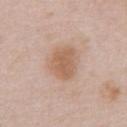Background:
About 4 mm across. A male patient approximately 55 years of age. The lesion is on the abdomen. Captured under white-light illumination. The total-body-photography lesion software estimated a lesion area of about 10 mm², an outline eccentricity of about 0.65 (0 = round, 1 = elongated), and a shape-asymmetry score of about 0.2 (0 = symmetric). It also reported an automated nevus-likeness rating near 50 out of 100 and lesion-presence confidence of about 100/100. This image is a 15 mm lesion crop taken from a total-body photograph.